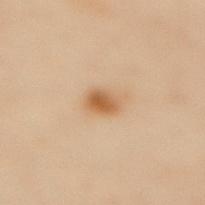Impression:
No biopsy was performed on this lesion — it was imaged during a full skin examination and was not determined to be concerning.
Clinical summary:
A close-up tile cropped from a whole-body skin photograph, about 15 mm across. A female subject, aged around 60. The recorded lesion diameter is about 2.5 mm. Imaged with cross-polarized lighting. On the back.